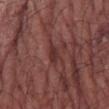The lesion was photographed on a routine skin check and not biopsied; there is no pathology result. The lesion-visualizer software estimated internal color variation of about 1.5 on a 0–10 scale and peripheral color asymmetry of about 0.5. It also reported a classifier nevus-likeness of about 0/100 and a lesion-detection confidence of about 70/100. Longest diameter approximately 3 mm. A region of skin cropped from a whole-body photographic capture, roughly 15 mm wide. Captured under white-light illumination. The lesion is located on the right forearm. A male subject, in their mid- to late 70s.